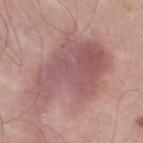Recorded during total-body skin imaging; not selected for excision or biopsy.
An algorithmic analysis of the crop reported a footprint of about 43 mm² and a shape eccentricity near 0.85. And it measured border irregularity of about 5 on a 0–10 scale, a within-lesion color-variation index near 4.5/10, and radial color variation of about 1.5.
The lesion is located on the left thigh.
A lesion tile, about 15 mm wide, cut from a 3D total-body photograph.
About 11 mm across.
A male patient aged around 50.